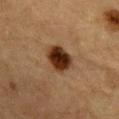Q: Was a biopsy performed?
A: no biopsy performed (imaged during a skin exam)
Q: Who is the patient?
A: male, approximately 85 years of age or older
Q: Where on the body is the lesion?
A: the front of the torso
Q: What is the imaging modality?
A: ~15 mm tile from a whole-body skin photo
Q: Lesion size?
A: about 4 mm